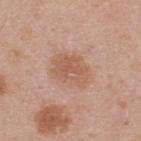Case summary:
• acquisition · total-body-photography crop, ~15 mm field of view
• lesion diameter · about 4.5 mm
• body site · the upper back
• subject · male, in their mid- to late 40s
• illumination · white-light